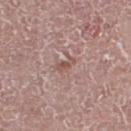The lesion was tiled from a total-body skin photograph and was not biopsied. A female subject about 65 years old. Imaged with white-light lighting. Longest diameter approximately 2.5 mm. An algorithmic analysis of the crop reported a lesion color around L≈53 a*≈20 b*≈23 in CIELAB, about 8 CIELAB-L* units darker than the surrounding skin, and a normalized border contrast of about 6.5. And it measured border irregularity of about 3.5 on a 0–10 scale, internal color variation of about 0.5 on a 0–10 scale, and radial color variation of about 0. The analysis additionally found a classifier nevus-likeness of about 0/100 and a detector confidence of about 95 out of 100 that the crop contains a lesion. Cropped from a whole-body photographic skin survey; the tile spans about 15 mm. Located on the right thigh.